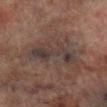image=~15 mm crop, total-body skin-cancer survey; tile lighting=cross-polarized; diameter=about 7 mm; patient=male, aged approximately 75; anatomic site=the right lower leg.